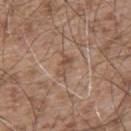Findings:
– notes · imaged on a skin check; not biopsied
– subject · male, aged approximately 55
– acquisition · total-body-photography crop, ~15 mm field of view
– size · about 3 mm
– lighting · white-light
– body site · the upper back